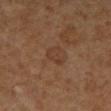Q: Was a biopsy performed?
A: no biopsy performed (imaged during a skin exam)
Q: What is the lesion's diameter?
A: ≈2.5 mm
Q: Lesion location?
A: the right lower leg
Q: What are the patient's age and sex?
A: female, aged approximately 65
Q: What did automated image analysis measure?
A: a mean CIELAB color near L≈37 a*≈19 b*≈28, roughly 6 lightness units darker than nearby skin, and a normalized border contrast of about 5.5
Q: What is the imaging modality?
A: total-body-photography crop, ~15 mm field of view
Q: How was the tile lit?
A: cross-polarized